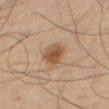notes=catalogued during a skin exam; not biopsied | illumination=cross-polarized | subject=male, approximately 65 years of age | diameter=about 3.5 mm | image=~15 mm crop, total-body skin-cancer survey | body site=the right thigh.